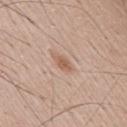Q: Was a biopsy performed?
A: imaged on a skin check; not biopsied
Q: What are the patient's age and sex?
A: male, aged 53–57
Q: What is the imaging modality?
A: ~15 mm crop, total-body skin-cancer survey
Q: Automated lesion metrics?
A: a lesion color around L≈58 a*≈20 b*≈30 in CIELAB, a lesion–skin lightness drop of about 9, and a lesion-to-skin contrast of about 7 (normalized; higher = more distinct); border irregularity of about 2 on a 0–10 scale, a within-lesion color-variation index near 1/10, and peripheral color asymmetry of about 0.5; a nevus-likeness score of about 50/100 and a lesion-detection confidence of about 100/100
Q: Lesion size?
A: ≈2.5 mm
Q: What lighting was used for the tile?
A: white-light illumination